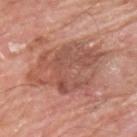biopsy status = total-body-photography surveillance lesion; no biopsy
patient = male, in their mid-60s
lesion diameter = about 8.5 mm
image = ~15 mm crop, total-body skin-cancer survey
tile lighting = white-light
anatomic site = the upper back